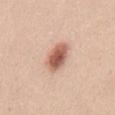Impression:
The lesion was tiled from a total-body skin photograph and was not biopsied.
Clinical summary:
A 15 mm close-up extracted from a 3D total-body photography capture. The subject is a male roughly 45 years of age. The lesion is located on the abdomen.